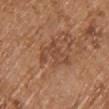Findings:
• workup — total-body-photography surveillance lesion; no biopsy
• site — the upper back
• lesion size — about 5 mm
• illumination — white-light
• image source — total-body-photography crop, ~15 mm field of view
• patient — male, roughly 70 years of age